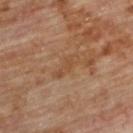The lesion was photographed on a routine skin check and not biopsied; there is no pathology result. Captured under cross-polarized illumination. The lesion's longest dimension is about 3.5 mm. The total-body-photography lesion software estimated an eccentricity of roughly 0.95. The software also gave a border-irregularity rating of about 4/10 and a within-lesion color-variation index near 1/10. A close-up tile cropped from a whole-body skin photograph, about 15 mm across. From the upper back. A male subject, aged approximately 85 or older.